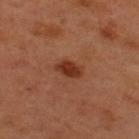Part of a total-body skin-imaging series; this lesion was reviewed on a skin check and was not flagged for biopsy. Automated image analysis of the tile measured about 10 CIELAB-L* units darker than the surrounding skin and a normalized lesion–skin contrast near 9. And it measured a peripheral color-asymmetry measure near 0.5. A male patient, about 50 years old. The lesion is on the upper back. This is a cross-polarized tile. This image is a 15 mm lesion crop taken from a total-body photograph.